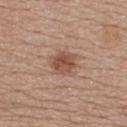Q: Was this lesion biopsied?
A: imaged on a skin check; not biopsied
Q: What kind of image is this?
A: 15 mm crop, total-body photography
Q: Lesion location?
A: the back
Q: Patient demographics?
A: female, aged approximately 60
Q: How large is the lesion?
A: ≈3 mm
Q: How was the tile lit?
A: white-light